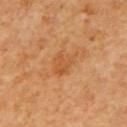Findings:
• notes: catalogued during a skin exam; not biopsied
• image source: total-body-photography crop, ~15 mm field of view
• subject: female
• site: the chest
• lighting: cross-polarized
• lesion size: ~3 mm (longest diameter)